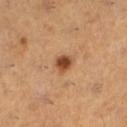  patient:
    sex: female
    age_approx: 55
  site: left lower leg
  lighting: cross-polarized
  image:
    source: total-body photography crop
    field_of_view_mm: 15
  lesion_size:
    long_diameter_mm_approx: 2.5